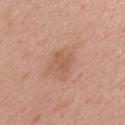– follow-up · no biopsy performed (imaged during a skin exam)
– illumination · white-light
– image · ~15 mm tile from a whole-body skin photo
– body site · the upper back
– automated metrics · a lesion area of about 4 mm², an outline eccentricity of about 0.75 (0 = round, 1 = elongated), and two-axis asymmetry of about 0.3; a classifier nevus-likeness of about 5/100 and a lesion-detection confidence of about 100/100
– lesion diameter · ~3 mm (longest diameter)
– patient · male, in their mid- to late 50s